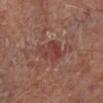Assessment:
Imaged during a routine full-body skin examination; the lesion was not biopsied and no histopathology is available.
Image and clinical context:
The patient is a male roughly 65 years of age. Located on the left lower leg. The lesion-visualizer software estimated a lesion area of about 8 mm² and a symmetry-axis asymmetry near 0.3. The software also gave about 7 CIELAB-L* units darker than the surrounding skin and a normalized border contrast of about 6.5. The software also gave a border-irregularity rating of about 3.5/10 and a within-lesion color-variation index near 4.5/10. And it measured a detector confidence of about 100 out of 100 that the crop contains a lesion. A roughly 15 mm field-of-view crop from a total-body skin photograph. Longest diameter approximately 4 mm. The tile uses cross-polarized illumination.